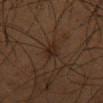biopsy_status: not biopsied; imaged during a skin examination
patient:
  sex: male
  age_approx: 55
site: abdomen
image:
  source: total-body photography crop
  field_of_view_mm: 15